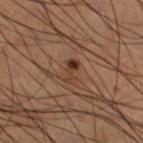Imaged during a routine full-body skin examination; the lesion was not biopsied and no histopathology is available.
A male patient, aged around 65.
The lesion is located on the left lower leg.
A 15 mm close-up tile from a total-body photography series done for melanoma screening.
Measured at roughly 3 mm in maximum diameter.